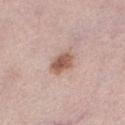notes: imaged on a skin check; not biopsied
patient: female, in their mid-60s
diameter: ~3.5 mm (longest diameter)
image: ~15 mm crop, total-body skin-cancer survey
location: the left lower leg
lighting: white-light
TBP lesion metrics: about 13 CIELAB-L* units darker than the surrounding skin and a normalized border contrast of about 9; a border-irregularity index near 2.5/10, a color-variation rating of about 3.5/10, and a peripheral color-asymmetry measure near 1